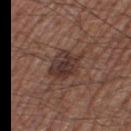Q: Was a biopsy performed?
A: no biopsy performed (imaged during a skin exam)
Q: How was this image acquired?
A: total-body-photography crop, ~15 mm field of view
Q: Where on the body is the lesion?
A: the right thigh
Q: What are the patient's age and sex?
A: male, roughly 55 years of age
Q: What did automated image analysis measure?
A: an average lesion color of about L≈35 a*≈18 b*≈22 (CIELAB) and a normalized border contrast of about 8.5; a border-irregularity index near 3/10, a color-variation rating of about 4.5/10, and radial color variation of about 1.5; lesion-presence confidence of about 100/100